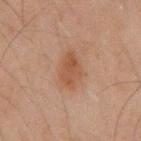Assessment:
The lesion was tiled from a total-body skin photograph and was not biopsied.
Context:
A male patient, roughly 45 years of age. This is a cross-polarized tile. A lesion tile, about 15 mm wide, cut from a 3D total-body photograph. Automated tile analysis of the lesion measured an eccentricity of roughly 0.75 and two-axis asymmetry of about 0.2. It also reported a mean CIELAB color near L≈42 a*≈18 b*≈27 and roughly 7 lightness units darker than nearby skin. And it measured an automated nevus-likeness rating near 65 out of 100 and lesion-presence confidence of about 100/100. From the mid back. Longest diameter approximately 4 mm.